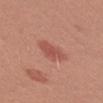Notes:
• workup · no biopsy performed (imaged during a skin exam)
• diameter · ≈3.5 mm
• image-analysis metrics · an area of roughly 7 mm², a shape eccentricity near 0.75, and a symmetry-axis asymmetry near 0.3
• imaging modality · ~15 mm crop, total-body skin-cancer survey
• location · the left forearm
• subject · female, roughly 35 years of age
• lighting · white-light illumination